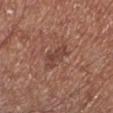Findings:
• biopsy status — imaged on a skin check; not biopsied
• subject — male, aged 68–72
• tile lighting — white-light illumination
• TBP lesion metrics — a lesion color around L≈43 a*≈22 b*≈26 in CIELAB and a lesion-to-skin contrast of about 6.5 (normalized; higher = more distinct); a border-irregularity rating of about 4.5/10, a within-lesion color-variation index near 2/10, and a peripheral color-asymmetry measure near 0.5; a nevus-likeness score of about 0/100 and a lesion-detection confidence of about 100/100
• image — 15 mm crop, total-body photography
• lesion diameter — ≈3.5 mm
• site — the left lower leg